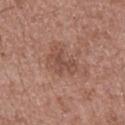Part of a total-body skin-imaging series; this lesion was reviewed on a skin check and was not flagged for biopsy.